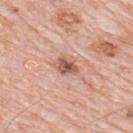lesion size: ≈3 mm | location: the upper back | imaging modality: ~15 mm crop, total-body skin-cancer survey | lighting: white-light | subject: male, about 80 years old.